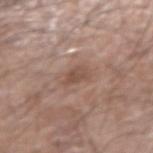{"biopsy_status": "not biopsied; imaged during a skin examination", "site": "right forearm", "image": {"source": "total-body photography crop", "field_of_view_mm": 15}, "patient": {"sex": "male", "age_approx": 80}, "lesion_size": {"long_diameter_mm_approx": 2.5}, "lighting": "white-light"}